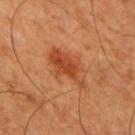<tbp_lesion>
  <biopsy_status>not biopsied; imaged during a skin examination</biopsy_status>
  <site>right upper arm</site>
  <lesion_size>
    <long_diameter_mm_approx>5.5</long_diameter_mm_approx>
  </lesion_size>
  <lighting>cross-polarized</lighting>
  <patient>
    <sex>male</sex>
    <age_approx>50</age_approx>
  </patient>
  <image>
    <source>total-body photography crop</source>
    <field_of_view_mm>15</field_of_view_mm>
  </image>
  <automated_metrics>
    <border_irregularity_0_10>4.0</border_irregularity_0_10>
    <color_variation_0_10>5.0</color_variation_0_10>
  </automated_metrics>
</tbp_lesion>